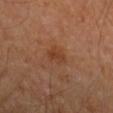Clinical impression:
No biopsy was performed on this lesion — it was imaged during a full skin examination and was not determined to be concerning.
Acquisition and patient details:
A male subject approximately 65 years of age. A close-up tile cropped from a whole-body skin photograph, about 15 mm across. The lesion is located on the arm.